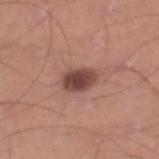notes=catalogued during a skin exam; not biopsied
anatomic site=the leg
subject=male, in their 30s
image source=~15 mm crop, total-body skin-cancer survey